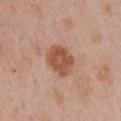follow-up: imaged on a skin check; not biopsied | imaging modality: ~15 mm tile from a whole-body skin photo | site: the chest | subject: female, in their mid- to late 60s.